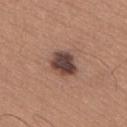Case summary:
- workup — no biopsy performed (imaged during a skin exam)
- patient — male, in their mid- to late 60s
- body site — the lower back
- acquisition — ~15 mm tile from a whole-body skin photo
- automated lesion analysis — a lesion area of about 8 mm², an eccentricity of roughly 0.55, and a shape-asymmetry score of about 0.2 (0 = symmetric); an average lesion color of about L≈42 a*≈18 b*≈22 (CIELAB), about 16 CIELAB-L* units darker than the surrounding skin, and a lesion-to-skin contrast of about 12.5 (normalized; higher = more distinct)